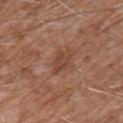– biopsy status — imaged on a skin check; not biopsied
– lesion diameter — about 3 mm
– patient — male, in their 60s
– illumination — white-light illumination
– location — the upper back
– image source — total-body-photography crop, ~15 mm field of view